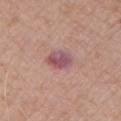| feature | finding |
|---|---|
| follow-up | no biopsy performed (imaged during a skin exam) |
| lighting | white-light |
| image | total-body-photography crop, ~15 mm field of view |
| automated metrics | a mean CIELAB color near L≈53 a*≈25 b*≈17, roughly 11 lightness units darker than nearby skin, and a normalized border contrast of about 9; a border-irregularity index near 1.5/10, a within-lesion color-variation index near 5/10, and peripheral color asymmetry of about 1.5 |
| anatomic site | the left upper arm |
| patient | male, aged 73 to 77 |